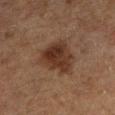| field | value |
|---|---|
| workup | imaged on a skin check; not biopsied |
| acquisition | ~15 mm crop, total-body skin-cancer survey |
| anatomic site | the left lower leg |
| subject | male, aged approximately 75 |